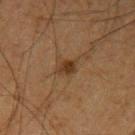Q: Was a biopsy performed?
A: total-body-photography surveillance lesion; no biopsy
Q: Illumination type?
A: cross-polarized
Q: Patient demographics?
A: male, in their mid- to late 60s
Q: What kind of image is this?
A: total-body-photography crop, ~15 mm field of view
Q: Lesion location?
A: the left upper arm